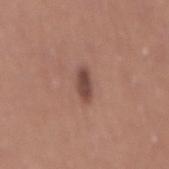lesion size: about 3.5 mm; body site: the back; imaging modality: ~15 mm tile from a whole-body skin photo; image-analysis metrics: a nevus-likeness score of about 90/100 and lesion-presence confidence of about 100/100; lighting: white-light illumination; subject: female, roughly 50 years of age.